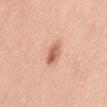Assessment: The lesion was photographed on a routine skin check and not biopsied; there is no pathology result. Background: The lesion-visualizer software estimated an area of roughly 5.5 mm², an outline eccentricity of about 0.9 (0 = round, 1 = elongated), and two-axis asymmetry of about 0.15. It also reported an average lesion color of about L≈63 a*≈24 b*≈33 (CIELAB), about 12 CIELAB-L* units darker than the surrounding skin, and a normalized border contrast of about 7.5. The analysis additionally found border irregularity of about 2 on a 0–10 scale, a color-variation rating of about 6/10, and a peripheral color-asymmetry measure near 2.5. The software also gave lesion-presence confidence of about 100/100. From the abdomen. A female patient, aged approximately 50. This is a white-light tile. A 15 mm close-up tile from a total-body photography series done for melanoma screening.